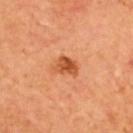follow-up = total-body-photography surveillance lesion; no biopsy
lesion diameter = ~3 mm (longest diameter)
body site = the upper back
tile lighting = cross-polarized illumination
subject = male, in their mid-60s
image = total-body-photography crop, ~15 mm field of view